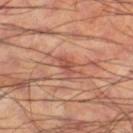Part of a total-body skin-imaging series; this lesion was reviewed on a skin check and was not flagged for biopsy. Cropped from a total-body skin-imaging series; the visible field is about 15 mm. The subject is a male approximately 60 years of age. On the right thigh.